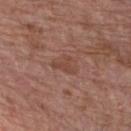Recorded during total-body skin imaging; not selected for excision or biopsy.
Located on the chest.
The subject is a male aged 58 to 62.
A region of skin cropped from a whole-body photographic capture, roughly 15 mm wide.